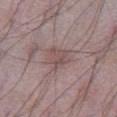follow-up: imaged on a skin check; not biopsied
image: ~15 mm tile from a whole-body skin photo
diameter: about 3 mm
patient: male, about 65 years old
anatomic site: the front of the torso
automated lesion analysis: an area of roughly 7.5 mm², a shape eccentricity near 0.4, and a shape-asymmetry score of about 0.3 (0 = symmetric); an average lesion color of about L≈50 a*≈17 b*≈18 (CIELAB)